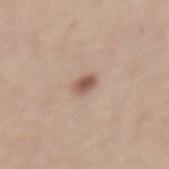Findings:
* notes: total-body-photography surveillance lesion; no biopsy
* image-analysis metrics: a lesion area of about 3.5 mm², an eccentricity of roughly 0.8, and a shape-asymmetry score of about 0.2 (0 = symmetric); an average lesion color of about L≈55 a*≈19 b*≈26 (CIELAB); a classifier nevus-likeness of about 90/100
* lighting: white-light illumination
* acquisition: ~15 mm crop, total-body skin-cancer survey
* body site: the back
* lesion size: ~2.5 mm (longest diameter)
* patient: female, about 65 years old